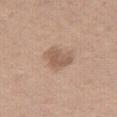Part of a total-body skin-imaging series; this lesion was reviewed on a skin check and was not flagged for biopsy. A male patient, approximately 65 years of age. The recorded lesion diameter is about 3.5 mm. Captured under white-light illumination. A 15 mm crop from a total-body photograph taken for skin-cancer surveillance. Located on the leg.